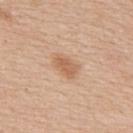Q: Was this lesion biopsied?
A: no biopsy performed (imaged during a skin exam)